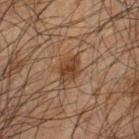{"image": {"source": "total-body photography crop", "field_of_view_mm": 15}, "site": "left forearm", "patient": {"sex": "male", "age_approx": 65}}